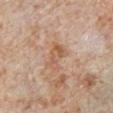Assessment: Recorded during total-body skin imaging; not selected for excision or biopsy. Image and clinical context: On the right lower leg. A female patient aged approximately 50. This image is a 15 mm lesion crop taken from a total-body photograph. This is a cross-polarized tile. About 4 mm across.